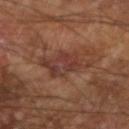size = ~5 mm (longest diameter) | subject = male, aged approximately 65 | anatomic site = the left arm | acquisition = ~15 mm crop, total-body skin-cancer survey | automated metrics = a lesion area of about 12 mm² and a symmetry-axis asymmetry near 0.3; a mean CIELAB color near L≈36 a*≈21 b*≈25 and a normalized lesion–skin contrast near 7; border irregularity of about 4.5 on a 0–10 scale, internal color variation of about 5 on a 0–10 scale, and peripheral color asymmetry of about 2; a classifier nevus-likeness of about 0/100 | tile lighting = cross-polarized illumination.